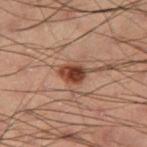notes = catalogued during a skin exam; not biopsied | size = ~3 mm (longest diameter) | subject = male, about 55 years old | location = the right thigh | image source = total-body-photography crop, ~15 mm field of view.